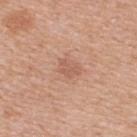Q: Is there a histopathology result?
A: no biopsy performed (imaged during a skin exam)
Q: How was this image acquired?
A: total-body-photography crop, ~15 mm field of view
Q: Where on the body is the lesion?
A: the upper back
Q: What are the patient's age and sex?
A: female, aged around 40